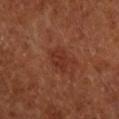Clinical impression: Imaged during a routine full-body skin examination; the lesion was not biopsied and no histopathology is available. Background: Measured at roughly 3 mm in maximum diameter. The lesion is located on the right forearm. The patient is a female aged 63 to 67. The total-body-photography lesion software estimated a symmetry-axis asymmetry near 0.2. The software also gave an automated nevus-likeness rating near 5 out of 100 and a lesion-detection confidence of about 100/100. A 15 mm crop from a total-body photograph taken for skin-cancer surveillance. Captured under cross-polarized illumination.